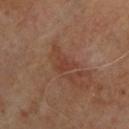Imaged during a routine full-body skin examination; the lesion was not biopsied and no histopathology is available. From the back. A lesion tile, about 15 mm wide, cut from a 3D total-body photograph. The tile uses cross-polarized illumination. A male subject roughly 65 years of age. About 3 mm across.